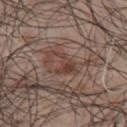workup: imaged on a skin check; not biopsied | image-analysis metrics: an area of roughly 5 mm² and a shape-asymmetry score of about 0.65 (0 = symmetric) | patient: male, roughly 50 years of age | lighting: white-light illumination | image source: ~15 mm tile from a whole-body skin photo | lesion diameter: about 4 mm | location: the chest.